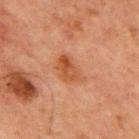Captured during whole-body skin photography for melanoma surveillance; the lesion was not biopsied. Captured under cross-polarized illumination. The lesion is located on the chest. A male subject aged approximately 65. A 15 mm crop from a total-body photograph taken for skin-cancer surveillance.